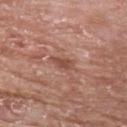  biopsy_status: not biopsied; imaged during a skin examination
  patient:
    sex: male
    age_approx: 65
  image:
    source: total-body photography crop
    field_of_view_mm: 15
  site: chest